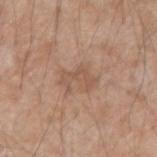No biopsy was performed on this lesion — it was imaged during a full skin examination and was not determined to be concerning.
Imaged with white-light lighting.
The lesion is on the right forearm.
The patient is a male approximately 70 years of age.
Approximately 3.5 mm at its widest.
A 15 mm close-up extracted from a 3D total-body photography capture.